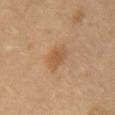A 15 mm crop from a total-body photograph taken for skin-cancer surveillance. Imaged with cross-polarized lighting. A female subject, about 55 years old. The lesion is located on the chest. The lesion's longest dimension is about 3.5 mm.